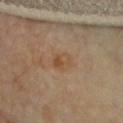Clinical impression: Part of a total-body skin-imaging series; this lesion was reviewed on a skin check and was not flagged for biopsy. Background: A 15 mm close-up extracted from a 3D total-body photography capture. A female subject in their 60s. From the chest.